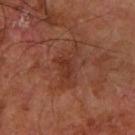Q: Was a biopsy performed?
A: catalogued during a skin exam; not biopsied
Q: Automated lesion metrics?
A: a lesion color around L≈34 a*≈24 b*≈28 in CIELAB, a lesion–skin lightness drop of about 6, and a lesion-to-skin contrast of about 6 (normalized; higher = more distinct); a border-irregularity rating of about 5.5/10 and a peripheral color-asymmetry measure near 1; a classifier nevus-likeness of about 0/100 and a detector confidence of about 100 out of 100 that the crop contains a lesion
Q: Patient demographics?
A: male, in their 60s
Q: What kind of image is this?
A: total-body-photography crop, ~15 mm field of view
Q: What is the anatomic site?
A: the arm
Q: What lighting was used for the tile?
A: cross-polarized illumination
Q: What is the lesion's diameter?
A: about 5 mm